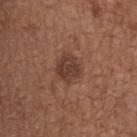Case summary:
* biopsy status · total-body-photography surveillance lesion; no biopsy
* lesion size · ≈3 mm
* patient · female, in their mid-60s
* tile lighting · white-light
* site · the back
* image source · total-body-photography crop, ~15 mm field of view